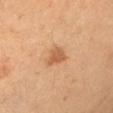Part of a total-body skin-imaging series; this lesion was reviewed on a skin check and was not flagged for biopsy. The lesion is located on the chest. Longest diameter approximately 3 mm. Captured under cross-polarized illumination. Cropped from a total-body skin-imaging series; the visible field is about 15 mm. A male patient, aged 58 to 62.